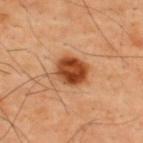notes: no biopsy performed (imaged during a skin exam) | location: the upper back | image-analysis metrics: about 18 CIELAB-L* units darker than the surrounding skin; a border-irregularity index near 1/10 and internal color variation of about 6 on a 0–10 scale | size: about 3.5 mm | imaging modality: 15 mm crop, total-body photography | tile lighting: cross-polarized | subject: male, about 40 years old.